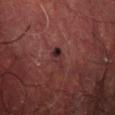A region of skin cropped from a whole-body photographic capture, roughly 15 mm wide.
The lesion is on the left thigh.
A male subject, aged approximately 60.
Measured at roughly 2.5 mm in maximum diameter.
The tile uses cross-polarized illumination.